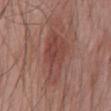Background:
From the front of the torso. A 15 mm crop from a total-body photograph taken for skin-cancer surveillance. The subject is a male in their 70s.